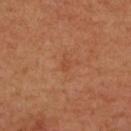| key | value |
|---|---|
| notes | imaged on a skin check; not biopsied |
| imaging modality | ~15 mm tile from a whole-body skin photo |
| site | the upper back |
| automated metrics | a lesion area of about 1.5 mm² and a shape-asymmetry score of about 0.25 (0 = symmetric); a within-lesion color-variation index near 0/10 and peripheral color asymmetry of about 0 |
| patient | male, in their mid-60s |